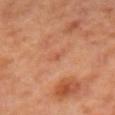A region of skin cropped from a whole-body photographic capture, roughly 15 mm wide. The subject is a female aged 48–52. The lesion-visualizer software estimated a symmetry-axis asymmetry near 0.45. The software also gave an average lesion color of about L≈52 a*≈28 b*≈35 (CIELAB), a lesion–skin lightness drop of about 6, and a normalized lesion–skin contrast near 5. And it measured border irregularity of about 3.5 on a 0–10 scale, a within-lesion color-variation index near 0/10, and a peripheral color-asymmetry measure near 0. The lesion is located on the left forearm. Measured at roughly 1 mm in maximum diameter.